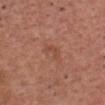Notes:
• biopsy status: no biopsy performed (imaged during a skin exam)
• location: the chest
• image source: ~15 mm tile from a whole-body skin photo
• automated metrics: a footprint of about 4 mm², a shape eccentricity near 0.85, and a symmetry-axis asymmetry near 0.4; about 6 CIELAB-L* units darker than the surrounding skin and a lesion-to-skin contrast of about 4.5 (normalized; higher = more distinct); a border-irregularity rating of about 4/10, internal color variation of about 1 on a 0–10 scale, and radial color variation of about 0
• lighting: white-light illumination
• patient: male, aged around 65
• lesion size: about 3 mm